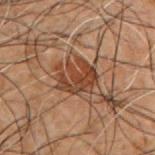{
  "biopsy_status": "not biopsied; imaged during a skin examination",
  "patient": {
    "sex": "male",
    "age_approx": 50
  },
  "lesion_size": {
    "long_diameter_mm_approx": 4.0
  },
  "lighting": "cross-polarized",
  "automated_metrics": {
    "border_irregularity_0_10": 5.5,
    "color_variation_0_10": 2.5,
    "nevus_likeness_0_100": 50,
    "lesion_detection_confidence_0_100": 95
  },
  "image": {
    "source": "total-body photography crop",
    "field_of_view_mm": 15
  },
  "site": "chest"
}